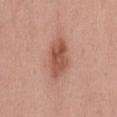Impression: No biopsy was performed on this lesion — it was imaged during a full skin examination and was not determined to be concerning. Clinical summary: On the back. A female patient, aged approximately 55. A roughly 15 mm field-of-view crop from a total-body skin photograph.